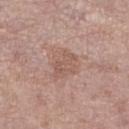Clinical impression:
Imaged during a routine full-body skin examination; the lesion was not biopsied and no histopathology is available.
Image and clinical context:
The lesion's longest dimension is about 4.5 mm. The lesion-visualizer software estimated an area of roughly 11 mm², a shape eccentricity near 0.6, and two-axis asymmetry of about 0.35. It also reported a mean CIELAB color near L≈57 a*≈19 b*≈25, a lesion–skin lightness drop of about 7, and a normalized lesion–skin contrast near 5. It also reported a border-irregularity index near 3.5/10 and a color-variation rating of about 3/10. A region of skin cropped from a whole-body photographic capture, roughly 15 mm wide. Imaged with white-light lighting. Located on the leg. A female subject aged 83 or over.